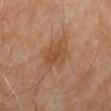Findings:
- notes: imaged on a skin check; not biopsied
- subject: male, in their 60s
- size: ~3.5 mm (longest diameter)
- image: ~15 mm tile from a whole-body skin photo
- TBP lesion metrics: an area of roughly 8.5 mm² and a shape eccentricity near 0.45; an average lesion color of about L≈46 a*≈20 b*≈32 (CIELAB), about 7 CIELAB-L* units darker than the surrounding skin, and a normalized lesion–skin contrast near 6; border irregularity of about 2 on a 0–10 scale and a color-variation rating of about 3/10
- body site: the lower back
- lighting: cross-polarized